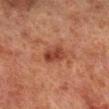Notes:
– biopsy status — total-body-photography surveillance lesion; no biopsy
– patient — male, in their 70s
– image source — 15 mm crop, total-body photography
– anatomic site — the right lower leg